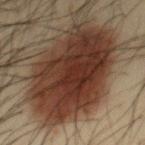The lesion was tiled from a total-body skin photograph and was not biopsied. From the mid back. The total-body-photography lesion software estimated an average lesion color of about L≈31 a*≈17 b*≈24 (CIELAB), about 15 CIELAB-L* units darker than the surrounding skin, and a normalized lesion–skin contrast near 13.5. The software also gave a border-irregularity index near 2.5/10, internal color variation of about 5.5 on a 0–10 scale, and radial color variation of about 2. The software also gave an automated nevus-likeness rating near 100 out of 100 and lesion-presence confidence of about 100/100. A lesion tile, about 15 mm wide, cut from a 3D total-body photograph. A male patient aged around 35. This is a cross-polarized tile. The lesion's longest dimension is about 12.5 mm.